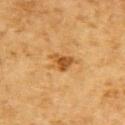Captured during whole-body skin photography for melanoma surveillance; the lesion was not biopsied.